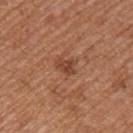Impression: Part of a total-body skin-imaging series; this lesion was reviewed on a skin check and was not flagged for biopsy. Acquisition and patient details: The lesion is on the left upper arm. Automated image analysis of the tile measured a mean CIELAB color near L≈44 a*≈24 b*≈32 and about 9 CIELAB-L* units darker than the surrounding skin. Captured under white-light illumination. Cropped from a whole-body photographic skin survey; the tile spans about 15 mm. The recorded lesion diameter is about 2.5 mm. A male patient, approximately 55 years of age.